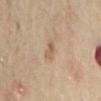Assessment:
No biopsy was performed on this lesion — it was imaged during a full skin examination and was not determined to be concerning.
Context:
The patient is a female about 60 years old. The lesion is on the lower back. A 15 mm close-up extracted from a 3D total-body photography capture.